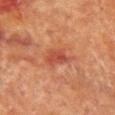  lesion_size:
    long_diameter_mm_approx: 3.5
  patient:
    sex: female
    age_approx: 80
  image:
    source: total-body photography crop
    field_of_view_mm: 15
  lighting: cross-polarized
  site: chest